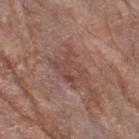  biopsy_status: not biopsied; imaged during a skin examination
  lesion_size:
    long_diameter_mm_approx: 4.0
  lighting: white-light
  patient:
    sex: female
    age_approx: 80
  image:
    source: total-body photography crop
    field_of_view_mm: 15
  site: right thigh
  automated_metrics:
    area_mm2_approx: 7.0
    eccentricity: 0.75
    shape_asymmetry: 0.35
    vs_skin_darker_L: 7.0
    vs_skin_contrast_norm: 5.5